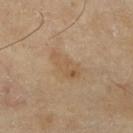The lesion was photographed on a routine skin check and not biopsied; there is no pathology result. A female patient, aged 68 to 72. A roughly 15 mm field-of-view crop from a total-body skin photograph. This is a cross-polarized tile. The lesion's longest dimension is about 4.5 mm. The lesion is on the right lower leg.